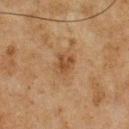The lesion was photographed on a routine skin check and not biopsied; there is no pathology result. The lesion is located on the front of the torso. Imaged with cross-polarized lighting. Automated tile analysis of the lesion measured a lesion color around L≈39 a*≈17 b*≈30 in CIELAB and a normalized border contrast of about 6.5. The analysis additionally found a border-irregularity index near 2.5/10 and a peripheral color-asymmetry measure near 1. About 3 mm across. A male subject, aged 73–77. A region of skin cropped from a whole-body photographic capture, roughly 15 mm wide.